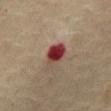<tbp_lesion>
<biopsy_status>not biopsied; imaged during a skin examination</biopsy_status>
<lesion_size>
  <long_diameter_mm_approx>3.5</long_diameter_mm_approx>
</lesion_size>
<patient>
  <sex>male</sex>
  <age_approx>75</age_approx>
</patient>
<site>abdomen</site>
<lighting>cross-polarized</lighting>
<image>
  <source>total-body photography crop</source>
  <field_of_view_mm>15</field_of_view_mm>
</image>
</tbp_lesion>